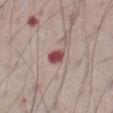biopsy_status: not biopsied; imaged during a skin examination
lesion_size:
  long_diameter_mm_approx: 2.5
automated_metrics:
  area_mm2_approx: 4.0
  eccentricity: 0.65
  shape_asymmetry: 0.3
  cielab_L: 49
  cielab_a: 29
  cielab_b: 20
  vs_skin_darker_L: 16.0
  border_irregularity_0_10: 2.5
  color_variation_0_10: 4.5
  peripheral_color_asymmetry: 1.5
lighting: white-light
site: abdomen
patient:
  sex: male
  age_approx: 75
image:
  source: total-body photography crop
  field_of_view_mm: 15